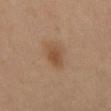Assessment: This lesion was catalogued during total-body skin photography and was not selected for biopsy. Context: An algorithmic analysis of the crop reported an average lesion color of about L≈49 a*≈18 b*≈32 (CIELAB), a lesion–skin lightness drop of about 8, and a lesion-to-skin contrast of about 7 (normalized; higher = more distinct). The analysis additionally found a border-irregularity index near 2.5/10, a color-variation rating of about 3/10, and peripheral color asymmetry of about 1. The analysis additionally found an automated nevus-likeness rating near 85 out of 100 and a detector confidence of about 100 out of 100 that the crop contains a lesion. This is a cross-polarized tile. The recorded lesion diameter is about 3.5 mm. A male subject, approximately 55 years of age. From the front of the torso. This image is a 15 mm lesion crop taken from a total-body photograph.